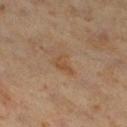Clinical impression: Part of a total-body skin-imaging series; this lesion was reviewed on a skin check and was not flagged for biopsy. Acquisition and patient details: From the right thigh. The tile uses cross-polarized illumination. About 3 mm across. The patient is a male roughly 60 years of age. A roughly 15 mm field-of-view crop from a total-body skin photograph. The total-body-photography lesion software estimated an area of roughly 4 mm² and a shape eccentricity near 0.75. The analysis additionally found an average lesion color of about L≈45 a*≈17 b*≈31 (CIELAB) and a normalized border contrast of about 6.